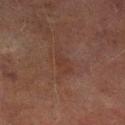The lesion was photographed on a routine skin check and not biopsied; there is no pathology result.